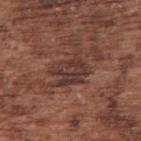Clinical impression:
The lesion was tiled from a total-body skin photograph and was not biopsied.
Image and clinical context:
Captured under white-light illumination. A male subject aged 73–77. Measured at roughly 5.5 mm in maximum diameter. A 15 mm close-up extracted from a 3D total-body photography capture. From the arm.